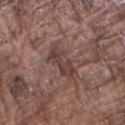Q: Was this lesion biopsied?
A: no biopsy performed (imaged during a skin exam)
Q: How was this image acquired?
A: total-body-photography crop, ~15 mm field of view
Q: Lesion location?
A: the right forearm
Q: Illumination type?
A: white-light illumination
Q: Who is the patient?
A: male, aged 73 to 77